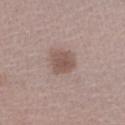follow-up = catalogued during a skin exam; not biopsied | site = the right forearm | acquisition = ~15 mm crop, total-body skin-cancer survey | subject = female, aged around 45.